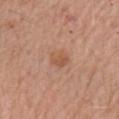biopsy_status: not biopsied; imaged during a skin examination
lesion_size:
  long_diameter_mm_approx: 2.5
automated_metrics:
  area_mm2_approx: 4.5
  eccentricity: 0.45
  shape_asymmetry: 0.2
  vs_skin_darker_L: 7.0
  vs_skin_contrast_norm: 5.5
image:
  source: total-body photography crop
  field_of_view_mm: 15
lighting: white-light
site: arm
patient:
  sex: female
  age_approx: 65